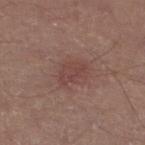follow-up: catalogued during a skin exam; not biopsied | body site: the left lower leg | diameter: ≈3.5 mm | acquisition: ~15 mm tile from a whole-body skin photo | automated metrics: an area of roughly 5.5 mm², an eccentricity of roughly 0.8, and two-axis asymmetry of about 0.3; a lesion color around L≈43 a*≈21 b*≈22 in CIELAB, a lesion–skin lightness drop of about 6, and a lesion-to-skin contrast of about 5 (normalized; higher = more distinct) | subject: male, approximately 45 years of age | tile lighting: white-light illumination.